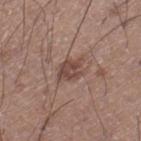Findings:
* biopsy status: catalogued during a skin exam; not biopsied
* TBP lesion metrics: a lesion area of about 5.5 mm² and a symmetry-axis asymmetry near 0.2; a border-irregularity index near 3/10; an automated nevus-likeness rating near 15 out of 100 and a lesion-detection confidence of about 100/100
* illumination: white-light illumination
* body site: the right lower leg
* patient: male, roughly 20 years of age
* image: ~15 mm tile from a whole-body skin photo
* diameter: ≈3 mm